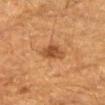Imaged during a routine full-body skin examination; the lesion was not biopsied and no histopathology is available.
The patient is a male aged approximately 85 or older.
The lesion is on the right upper arm.
A lesion tile, about 15 mm wide, cut from a 3D total-body photograph.